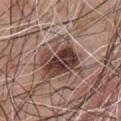Q: Was a biopsy performed?
A: total-body-photography surveillance lesion; no biopsy
Q: How large is the lesion?
A: ≈5.5 mm
Q: What kind of image is this?
A: ~15 mm tile from a whole-body skin photo
Q: What did automated image analysis measure?
A: a lesion–skin lightness drop of about 14 and a normalized border contrast of about 10.5; a border-irregularity index near 6/10, internal color variation of about 10 on a 0–10 scale, and a peripheral color-asymmetry measure near 3.5
Q: Patient demographics?
A: male, aged approximately 75
Q: What is the anatomic site?
A: the chest